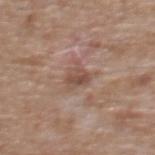Assessment:
Imaged during a routine full-body skin examination; the lesion was not biopsied and no histopathology is available.
Acquisition and patient details:
A roughly 15 mm field-of-view crop from a total-body skin photograph. A male patient aged approximately 60. The tile uses white-light illumination. Automated image analysis of the tile measured a lesion color around L≈50 a*≈18 b*≈26 in CIELAB and about 9 CIELAB-L* units darker than the surrounding skin. The software also gave a lesion-detection confidence of about 100/100. The lesion is on the chest. The recorded lesion diameter is about 3 mm.